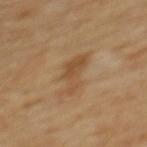A female subject approximately 60 years of age. This is a cross-polarized tile. A 15 mm crop from a total-body photograph taken for skin-cancer surveillance. The recorded lesion diameter is about 4.5 mm. From the upper back. An algorithmic analysis of the crop reported a footprint of about 9 mm², a shape eccentricity near 0.8, and a shape-asymmetry score of about 0.45 (0 = symmetric). And it measured border irregularity of about 5.5 on a 0–10 scale, a color-variation rating of about 4/10, and a peripheral color-asymmetry measure near 1.5.